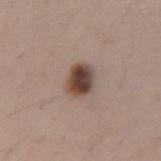Notes:
• notes — catalogued during a skin exam; not biopsied
• automated lesion analysis — a shape-asymmetry score of about 0.2 (0 = symmetric); a normalized lesion–skin contrast near 12; border irregularity of about 1.5 on a 0–10 scale, internal color variation of about 7 on a 0–10 scale, and peripheral color asymmetry of about 2; a classifier nevus-likeness of about 100/100 and a detector confidence of about 100 out of 100 that the crop contains a lesion
• anatomic site — the arm
• subject — female, in their mid-20s
• lesion diameter — ~3 mm (longest diameter)
• image source — ~15 mm crop, total-body skin-cancer survey